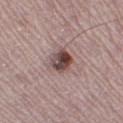workup = catalogued during a skin exam; not biopsied | imaging modality = total-body-photography crop, ~15 mm field of view | site = the right thigh | image-analysis metrics = an eccentricity of roughly 0.5 and a symmetry-axis asymmetry near 0.15; border irregularity of about 1.5 on a 0–10 scale and peripheral color asymmetry of about 3 | illumination = white-light | patient = female, approximately 50 years of age | lesion size = ≈3 mm.